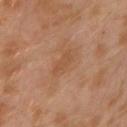Clinical summary: A male subject aged 28 to 32. Captured under cross-polarized illumination. A region of skin cropped from a whole-body photographic capture, roughly 15 mm wide. On the left arm.